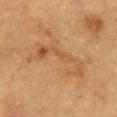| key | value |
|---|---|
| automated lesion analysis | a border-irregularity index near 10/10 and a color-variation rating of about 4.5/10; a detector confidence of about 100 out of 100 that the crop contains a lesion |
| subject | male, in their mid- to late 80s or older |
| image source | 15 mm crop, total-body photography |
| lesion size | ~7 mm (longest diameter) |
| illumination | cross-polarized |
| site | the front of the torso |